The patient is a female roughly 80 years of age · a 15 mm crop from a total-body photograph taken for skin-cancer surveillance · the lesion is on the left leg:
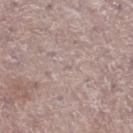diagnosis:
  histopathology: melanoma in situ
  malignancy: malignant
  taxonomic_path:
    - Malignant
    - Malignant melanocytic proliferations (Melanoma)
    - Melanoma in situ